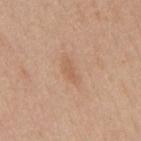This lesion was catalogued during total-body skin photography and was not selected for biopsy.
The tile uses white-light illumination.
The subject is a male aged 28 to 32.
A region of skin cropped from a whole-body photographic capture, roughly 15 mm wide.
The lesion is on the mid back.